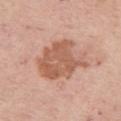Notes:
– notes: total-body-photography surveillance lesion; no biopsy
– image: total-body-photography crop, ~15 mm field of view
– lesion size: ≈6.5 mm
– lighting: white-light illumination
– image-analysis metrics: a footprint of about 21 mm², an eccentricity of roughly 0.65, and a shape-asymmetry score of about 0.3 (0 = symmetric); a border-irregularity index near 3.5/10, a color-variation rating of about 3/10, and peripheral color asymmetry of about 1
– anatomic site: the right thigh
– patient: female, aged 63 to 67